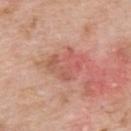{"lesion_size": {"long_diameter_mm_approx": 4.5}, "site": "back", "image": {"source": "total-body photography crop", "field_of_view_mm": 15}, "patient": {"sex": "male", "age_approx": 60}, "diagnosis": {"histopathology": "squamous cell carcinoma in situ", "malignancy": "malignant", "taxonomic_path": ["Malignant", "Malignant epidermal proliferations", "Squamous cell carcinoma in situ"]}}